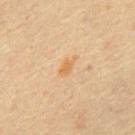This lesion was catalogued during total-body skin photography and was not selected for biopsy.
An algorithmic analysis of the crop reported a footprint of about 3.5 mm², a shape eccentricity near 0.9, and two-axis asymmetry of about 0.35. It also reported an average lesion color of about L≈60 a*≈16 b*≈39 (CIELAB), roughly 6 lightness units darker than nearby skin, and a normalized border contrast of about 6.5.
A male subject, aged 63 to 67.
Cropped from a whole-body photographic skin survey; the tile spans about 15 mm.
The recorded lesion diameter is about 3 mm.
On the chest.
This is a cross-polarized tile.